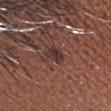notes = no biopsy performed (imaged during a skin exam).